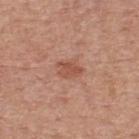Clinical impression: This lesion was catalogued during total-body skin photography and was not selected for biopsy. Context: Located on the back. Cropped from a total-body skin-imaging series; the visible field is about 15 mm. A male subject aged approximately 80. Measured at roughly 3 mm in maximum diameter.